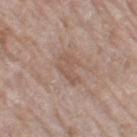{
  "biopsy_status": "not biopsied; imaged during a skin examination",
  "site": "left thigh",
  "automated_metrics": {
    "area_mm2_approx": 6.5,
    "eccentricity": 0.8,
    "shape_asymmetry": 0.4,
    "vs_skin_contrast_norm": 5.0,
    "border_irregularity_0_10": 4.5,
    "peripheral_color_asymmetry": 0.5
  },
  "image": {
    "source": "total-body photography crop",
    "field_of_view_mm": 15
  },
  "patient": {
    "sex": "female",
    "age_approx": 75
  },
  "lighting": "white-light",
  "lesion_size": {
    "long_diameter_mm_approx": 3.5
  }
}